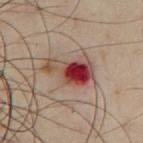The lesion was tiled from a total-body skin photograph and was not biopsied. From the chest. The patient is a male aged approximately 50. About 5.5 mm across. This is a cross-polarized tile. A 15 mm close-up extracted from a 3D total-body photography capture.